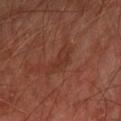Q: Was this lesion biopsied?
A: catalogued during a skin exam; not biopsied
Q: Patient demographics?
A: male, aged around 70
Q: What kind of image is this?
A: ~15 mm tile from a whole-body skin photo
Q: Where on the body is the lesion?
A: the left forearm
Q: How was the tile lit?
A: cross-polarized illumination
Q: Lesion size?
A: ≈4 mm